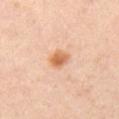The lesion was tiled from a total-body skin photograph and was not biopsied. This is a cross-polarized tile. The lesion's longest dimension is about 2.5 mm. Automated image analysis of the tile measured a color-variation rating of about 4/10 and a peripheral color-asymmetry measure near 1. The analysis additionally found a lesion-detection confidence of about 100/100. The subject is a female approximately 40 years of age. This image is a 15 mm lesion crop taken from a total-body photograph. The lesion is on the left thigh.